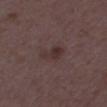Imaged during a routine full-body skin examination; the lesion was not biopsied and no histopathology is available. On the right thigh. A 15 mm close-up tile from a total-body photography series done for melanoma screening. Captured under white-light illumination. The subject is a female in their mid-30s.